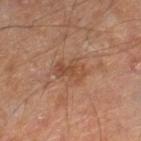{
  "biopsy_status": "not biopsied; imaged during a skin examination",
  "lighting": "cross-polarized",
  "site": "right lower leg",
  "patient": {
    "age_approx": 55
  },
  "image": {
    "source": "total-body photography crop",
    "field_of_view_mm": 15
  },
  "lesion_size": {
    "long_diameter_mm_approx": 3.5
  }
}